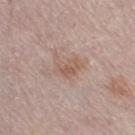Q: Was this lesion biopsied?
A: imaged on a skin check; not biopsied
Q: What is the lesion's diameter?
A: ~3.5 mm (longest diameter)
Q: What kind of image is this?
A: 15 mm crop, total-body photography
Q: Automated lesion metrics?
A: a lesion area of about 6 mm² and two-axis asymmetry of about 0.4; a border-irregularity rating of about 5/10 and internal color variation of about 3.5 on a 0–10 scale
Q: Where on the body is the lesion?
A: the left leg
Q: What lighting was used for the tile?
A: white-light
Q: What are the patient's age and sex?
A: female, roughly 65 years of age